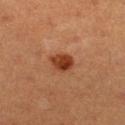Impression:
Imaged during a routine full-body skin examination; the lesion was not biopsied and no histopathology is available.
Clinical summary:
A female patient roughly 55 years of age. Cropped from a total-body skin-imaging series; the visible field is about 15 mm. On the right lower leg.